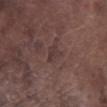| key | value |
|---|---|
| follow-up | catalogued during a skin exam; not biopsied |
| image | ~15 mm tile from a whole-body skin photo |
| illumination | white-light illumination |
| body site | the leg |
| subject | male, aged around 75 |